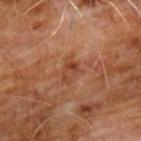Q: Was this lesion biopsied?
A: imaged on a skin check; not biopsied
Q: Patient demographics?
A: male, approximately 60 years of age
Q: Illumination type?
A: cross-polarized
Q: Where on the body is the lesion?
A: the chest
Q: What is the lesion's diameter?
A: ~3.5 mm (longest diameter)
Q: What kind of image is this?
A: 15 mm crop, total-body photography